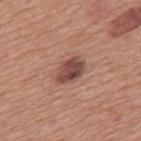This lesion was catalogued during total-body skin photography and was not selected for biopsy. A male subject, in their mid- to late 70s. About 4 mm across. The lesion is located on the mid back. A roughly 15 mm field-of-view crop from a total-body skin photograph.